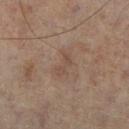tile lighting: cross-polarized
subject: male, roughly 65 years of age
site: the right lower leg
acquisition: total-body-photography crop, ~15 mm field of view